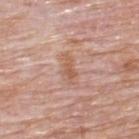Part of a total-body skin-imaging series; this lesion was reviewed on a skin check and was not flagged for biopsy.
A male patient approximately 75 years of age.
The lesion's longest dimension is about 2.5 mm.
The total-body-photography lesion software estimated a classifier nevus-likeness of about 0/100 and a detector confidence of about 100 out of 100 that the crop contains a lesion.
This is a white-light tile.
Located on the upper back.
A region of skin cropped from a whole-body photographic capture, roughly 15 mm wide.